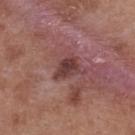notes: catalogued during a skin exam; not biopsied
tile lighting: white-light
subject: female, about 40 years old
body site: the upper back
acquisition: total-body-photography crop, ~15 mm field of view
lesion size: ~3.5 mm (longest diameter)